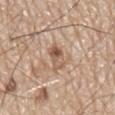biopsy status = total-body-photography surveillance lesion; no biopsy | body site = the mid back | subject = male, aged 78 to 82 | image = ~15 mm crop, total-body skin-cancer survey.